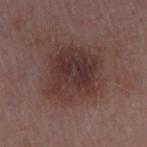Assessment:
Recorded during total-body skin imaging; not selected for excision or biopsy.